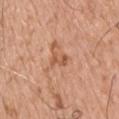• biopsy status · imaged on a skin check; not biopsied
• subject · male, in their mid- to late 70s
• image source · ~15 mm crop, total-body skin-cancer survey
• body site · the upper back